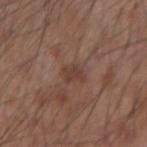| field | value |
|---|---|
| notes | no biopsy performed (imaged during a skin exam) |
| site | the left forearm |
| acquisition | ~15 mm crop, total-body skin-cancer survey |
| patient | male, approximately 55 years of age |